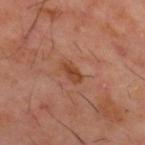Q: Is there a histopathology result?
A: no biopsy performed (imaged during a skin exam)
Q: How large is the lesion?
A: ≈3 mm
Q: What lighting was used for the tile?
A: cross-polarized illumination
Q: Where on the body is the lesion?
A: the mid back
Q: What are the patient's age and sex?
A: male, approximately 60 years of age
Q: How was this image acquired?
A: ~15 mm tile from a whole-body skin photo